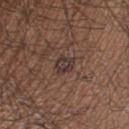biopsy status: catalogued during a skin exam; not biopsied | lighting: white-light | location: the right thigh | acquisition: ~15 mm crop, total-body skin-cancer survey | TBP lesion metrics: a mean CIELAB color near L≈34 a*≈15 b*≈19, about 8 CIELAB-L* units darker than the surrounding skin, and a lesion-to-skin contrast of about 8.5 (normalized; higher = more distinct); a nevus-likeness score of about 0/100 | diameter: about 2.5 mm | patient: male, aged around 35.